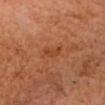{"biopsy_status": "not biopsied; imaged during a skin examination"}